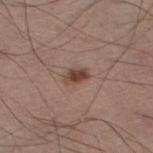{"biopsy_status": "not biopsied; imaged during a skin examination", "lesion_size": {"long_diameter_mm_approx": 2.5}, "site": "left thigh", "patient": {"sex": "male", "age_approx": 55}, "image": {"source": "total-body photography crop", "field_of_view_mm": 15}, "automated_metrics": {"area_mm2_approx": 4.0, "eccentricity": 0.8, "shape_asymmetry": 0.25, "cielab_L": 43, "cielab_a": 19, "cielab_b": 24, "vs_skin_darker_L": 11.0, "vs_skin_contrast_norm": 9.0, "nevus_likeness_0_100": 90}}